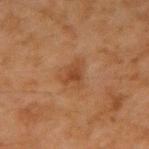Recorded during total-body skin imaging; not selected for excision or biopsy.
A male patient, aged approximately 45.
Captured under cross-polarized illumination.
Measured at roughly 3.5 mm in maximum diameter.
A region of skin cropped from a whole-body photographic capture, roughly 15 mm wide.
Located on the right upper arm.
The lesion-visualizer software estimated a lesion color around L≈37 a*≈19 b*≈30 in CIELAB, a lesion–skin lightness drop of about 7, and a normalized border contrast of about 6. And it measured a border-irregularity rating of about 5/10, internal color variation of about 3 on a 0–10 scale, and a peripheral color-asymmetry measure near 1. The software also gave a classifier nevus-likeness of about 0/100 and a detector confidence of about 100 out of 100 that the crop contains a lesion.